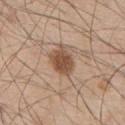Assessment:
The lesion was tiled from a total-body skin photograph and was not biopsied.
Image and clinical context:
Cropped from a total-body skin-imaging series; the visible field is about 15 mm. A male patient about 55 years old. From the upper back. This is a white-light tile.